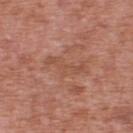Clinical impression: Part of a total-body skin-imaging series; this lesion was reviewed on a skin check and was not flagged for biopsy.